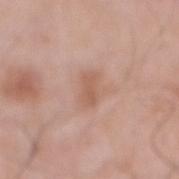biopsy status = total-body-photography surveillance lesion; no biopsy
lesion diameter = about 3 mm
subject = male, roughly 70 years of age
site = the abdomen
image = 15 mm crop, total-body photography
lighting = white-light